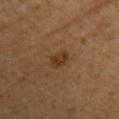Captured during whole-body skin photography for melanoma surveillance; the lesion was not biopsied. The lesion's longest dimension is about 3 mm. From the left upper arm. A roughly 15 mm field-of-view crop from a total-body skin photograph. The patient is a female in their 60s. Automated image analysis of the tile measured an area of roughly 4 mm² and a shape-asymmetry score of about 0.3 (0 = symmetric). It also reported roughly 8 lightness units darker than nearby skin.